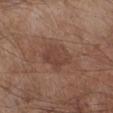follow-up: catalogued during a skin exam; not biopsied | illumination: white-light illumination | patient: male, in their mid- to late 50s | image: ~15 mm tile from a whole-body skin photo | automated lesion analysis: a lesion color around L≈42 a*≈20 b*≈25 in CIELAB, a lesion–skin lightness drop of about 7, and a normalized border contrast of about 6; a border-irregularity index near 3/10 and peripheral color asymmetry of about 1; a nevus-likeness score of about 5/100 | body site: the leg.